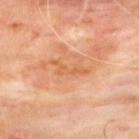{"biopsy_status": "not biopsied; imaged during a skin examination", "image": {"source": "total-body photography crop", "field_of_view_mm": 15}, "lesion_size": {"long_diameter_mm_approx": 4.5}, "patient": {"sex": "male", "age_approx": 65}, "site": "back"}